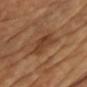– notes · imaged on a skin check; not biopsied
– size · ≈3.5 mm
– automated lesion analysis · a lesion area of about 8.5 mm², an eccentricity of roughly 0.45, and a shape-asymmetry score of about 0.35 (0 = symmetric); an average lesion color of about L≈41 a*≈22 b*≈33 (CIELAB), about 8 CIELAB-L* units darker than the surrounding skin, and a normalized border contrast of about 7; border irregularity of about 3.5 on a 0–10 scale and a peripheral color-asymmetry measure near 1
– acquisition · ~15 mm tile from a whole-body skin photo
– body site · the front of the torso
– illumination · cross-polarized illumination